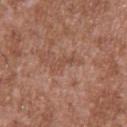Q: Is there a histopathology result?
A: catalogued during a skin exam; not biopsied
Q: Who is the patient?
A: male, aged around 45
Q: What is the anatomic site?
A: the upper back
Q: What is the imaging modality?
A: ~15 mm crop, total-body skin-cancer survey
Q: How large is the lesion?
A: ≈3 mm
Q: Illumination type?
A: white-light
Q: What did automated image analysis measure?
A: a lesion area of about 2.5 mm² and a shape eccentricity near 0.95; about 6 CIELAB-L* units darker than the surrounding skin and a normalized border contrast of about 4.5; border irregularity of about 5.5 on a 0–10 scale, internal color variation of about 0 on a 0–10 scale, and peripheral color asymmetry of about 0